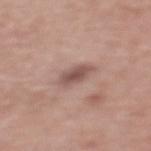  site: upper back
  automated_metrics:
    eccentricity: 0.8
    shape_asymmetry: 0.2
    cielab_L: 52
    cielab_a: 19
    cielab_b: 22
    vs_skin_darker_L: 11.0
    vs_skin_contrast_norm: 8.0
    border_irregularity_0_10: 2.0
    peripheral_color_asymmetry: 1.0
    nevus_likeness_0_100: 15
  lesion_size:
    long_diameter_mm_approx: 3.0
  lighting: white-light
  image:
    source: total-body photography crop
    field_of_view_mm: 15
  patient:
    sex: female
    age_approx: 70